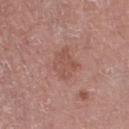The lesion was tiled from a total-body skin photograph and was not biopsied. A 15 mm close-up tile from a total-body photography series done for melanoma screening. An algorithmic analysis of the crop reported a lesion color around L≈52 a*≈23 b*≈26 in CIELAB, about 7 CIELAB-L* units darker than the surrounding skin, and a normalized border contrast of about 5. Located on the leg. A male patient aged 73–77. This is a white-light tile.